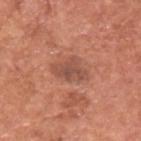{"image": {"source": "total-body photography crop", "field_of_view_mm": 15}, "site": "back", "patient": {"sex": "male", "age_approx": 65}}